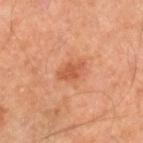Impression:
Captured during whole-body skin photography for melanoma surveillance; the lesion was not biopsied.